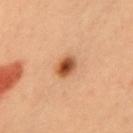This lesion was catalogued during total-body skin photography and was not selected for biopsy. A roughly 15 mm field-of-view crop from a total-body skin photograph. The lesion is located on the chest. Longest diameter approximately 3 mm. A female subject approximately 40 years of age.